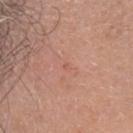diameter=≈1 mm
anatomic site=the head or neck
patient=female, approximately 35 years of age
acquisition=15 mm crop, total-body photography
tile lighting=white-light
TBP lesion metrics=an area of roughly 1 mm², a shape eccentricity near 0.65, and two-axis asymmetry of about 0.3; a mean CIELAB color near L≈57 a*≈25 b*≈29 and a normalized lesion–skin contrast near 3.5; a border-irregularity rating of about 2/10 and peripheral color asymmetry of about 0; a detector confidence of about 100 out of 100 that the crop contains a lesion
diagnosis=a superficial basal cell carcinoma — a skin cancer